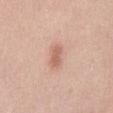follow-up = no biopsy performed (imaged during a skin exam)
acquisition = total-body-photography crop, ~15 mm field of view
lesion diameter = about 2.5 mm
patient = male, in their mid-50s
automated metrics = an eccentricity of roughly 0.85 and two-axis asymmetry of about 0.25; a mean CIELAB color near L≈62 a*≈23 b*≈29 and a lesion–skin lightness drop of about 10; a border-irregularity index near 2/10, a within-lesion color-variation index near 1/10, and peripheral color asymmetry of about 0.5; an automated nevus-likeness rating near 90 out of 100 and a lesion-detection confidence of about 100/100
lighting = white-light
body site = the abdomen